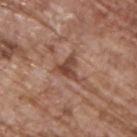Notes:
* follow-up: catalogued during a skin exam; not biopsied
* patient: male, in their 70s
* site: the upper back
* imaging modality: 15 mm crop, total-body photography